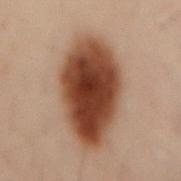Clinical impression:
The lesion was photographed on a routine skin check and not biopsied; there is no pathology result.
Background:
The patient is a male aged 48 to 52. A region of skin cropped from a whole-body photographic capture, roughly 15 mm wide. The lesion is on the lower back.